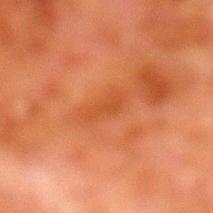Assessment:
The lesion was photographed on a routine skin check and not biopsied; there is no pathology result.
Clinical summary:
A male subject aged approximately 80. A 15 mm close-up tile from a total-body photography series done for melanoma screening. The lesion-visualizer software estimated an average lesion color of about L≈40 a*≈27 b*≈37 (CIELAB), roughly 5 lightness units darker than nearby skin, and a normalized lesion–skin contrast near 5. It also reported border irregularity of about 3.5 on a 0–10 scale. The analysis additionally found a classifier nevus-likeness of about 0/100 and lesion-presence confidence of about 100/100. On the left lower leg.